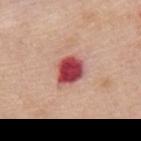No biopsy was performed on this lesion — it was imaged during a full skin examination and was not determined to be concerning.
A female subject in their mid- to late 60s.
Captured under white-light illumination.
About 4 mm across.
Located on the mid back.
A roughly 15 mm field-of-view crop from a total-body skin photograph.